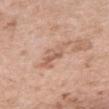Background:
About 5.5 mm across. A female subject in their 40s. The lesion is on the chest. The tile uses white-light illumination. A 15 mm crop from a total-body photograph taken for skin-cancer surveillance. Automated tile analysis of the lesion measured a shape eccentricity near 0.95 and a symmetry-axis asymmetry near 0.35.